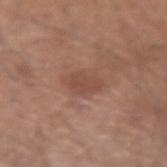<record>
<biopsy_status>not biopsied; imaged during a skin examination</biopsy_status>
<image>
  <source>total-body photography crop</source>
  <field_of_view_mm>15</field_of_view_mm>
</image>
<lesion_size>
  <long_diameter_mm_approx>3.5</long_diameter_mm_approx>
</lesion_size>
<site>arm</site>
<automated_metrics>
  <area_mm2_approx>6.5</area_mm2_approx>
  <eccentricity>0.75</eccentricity>
  <shape_asymmetry>0.3</shape_asymmetry>
  <cielab_L>48</cielab_L>
  <cielab_a>21</cielab_a>
  <cielab_b>27</cielab_b>
  <vs_skin_darker_L>7.0</vs_skin_darker_L>
  <vs_skin_contrast_norm>5.5</vs_skin_contrast_norm>
</automated_metrics>
<lighting>white-light</lighting>
<patient>
  <sex>male</sex>
  <age_approx>70</age_approx>
</patient>
</record>